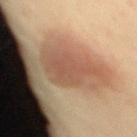Impression:
Recorded during total-body skin imaging; not selected for excision or biopsy.
Image and clinical context:
Located on the mid back. A female patient, aged around 35. Approximately 7 mm at its widest. A 15 mm close-up extracted from a 3D total-body photography capture.